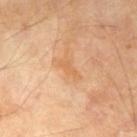Impression: This lesion was catalogued during total-body skin photography and was not selected for biopsy. Background: The lesion is on the left thigh. The subject is a male approximately 70 years of age. A region of skin cropped from a whole-body photographic capture, roughly 15 mm wide.